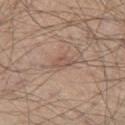Notes:
- biopsy status: imaged on a skin check; not biopsied
- image: ~15 mm crop, total-body skin-cancer survey
- patient: male, approximately 45 years of age
- body site: the left thigh
- size: ~3 mm (longest diameter)
- illumination: white-light